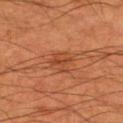{"biopsy_status": "not biopsied; imaged during a skin examination", "image": {"source": "total-body photography crop", "field_of_view_mm": 15}, "lesion_size": {"long_diameter_mm_approx": 3.0}, "automated_metrics": {"cielab_L": 45, "cielab_a": 28, "cielab_b": 37, "vs_skin_darker_L": 7.0, "vs_skin_contrast_norm": 5.5, "border_irregularity_0_10": 4.5, "color_variation_0_10": 3.5, "peripheral_color_asymmetry": 1.0}, "site": "left thigh", "patient": {"sex": "male", "age_approx": 55}}